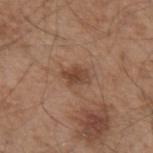Captured during whole-body skin photography for melanoma surveillance; the lesion was not biopsied.
A male subject, in their mid- to late 50s.
From the left upper arm.
A region of skin cropped from a whole-body photographic capture, roughly 15 mm wide.
Automated tile analysis of the lesion measured an area of roughly 4.5 mm² and two-axis asymmetry of about 0.35. And it measured a mean CIELAB color near L≈44 a*≈20 b*≈29, a lesion–skin lightness drop of about 10, and a normalized lesion–skin contrast near 8. And it measured a border-irregularity rating of about 3.5/10, internal color variation of about 3 on a 0–10 scale, and radial color variation of about 1. And it measured a nevus-likeness score of about 75/100 and a detector confidence of about 100 out of 100 that the crop contains a lesion.